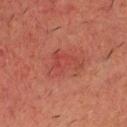<tbp_lesion>
  <site>head or neck</site>
  <image>
    <source>total-body photography crop</source>
    <field_of_view_mm>15</field_of_view_mm>
  </image>
  <patient>
    <sex>male</sex>
    <age_approx>65</age_approx>
  </patient>
</tbp_lesion>